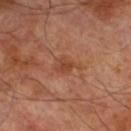Recorded during total-body skin imaging; not selected for excision or biopsy. A roughly 15 mm field-of-view crop from a total-body skin photograph. The lesion is on the left lower leg. The patient is a male aged approximately 70.